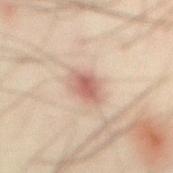This image is a 15 mm lesion crop taken from a total-body photograph.
The lesion is on the abdomen.
The total-body-photography lesion software estimated an average lesion color of about L≈54 a*≈20 b*≈24 (CIELAB), roughly 11 lightness units darker than nearby skin, and a normalized lesion–skin contrast near 7.5. The analysis additionally found border irregularity of about 2 on a 0–10 scale, internal color variation of about 3 on a 0–10 scale, and a peripheral color-asymmetry measure near 1. It also reported a nevus-likeness score of about 60/100 and a detector confidence of about 100 out of 100 that the crop contains a lesion.
Longest diameter approximately 3.5 mm.
This is a cross-polarized tile.
The subject is a male aged around 50.